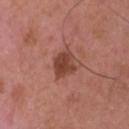This lesion was catalogued during total-body skin photography and was not selected for biopsy. The lesion-visualizer software estimated a border-irregularity rating of about 1.5/10, internal color variation of about 3 on a 0–10 scale, and peripheral color asymmetry of about 1. The software also gave a nevus-likeness score of about 85/100 and lesion-presence confidence of about 100/100. A male subject, aged around 50. From the head or neck. This image is a 15 mm lesion crop taken from a total-body photograph. The tile uses white-light illumination. Approximately 3 mm at its widest.